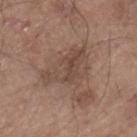  biopsy_status: not biopsied; imaged during a skin examination
  image:
    source: total-body photography crop
    field_of_view_mm: 15
  site: right lower leg
  patient:
    sex: male
    age_approx: 80